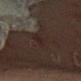Q: Was a biopsy performed?
A: imaged on a skin check; not biopsied
Q: Automated lesion metrics?
A: a footprint of about 3.5 mm², an eccentricity of roughly 0.8, and a shape-asymmetry score of about 0.3 (0 = symmetric); a lesion color around L≈19 a*≈12 b*≈14 in CIELAB, a lesion–skin lightness drop of about 5, and a normalized border contrast of about 6.5
Q: How was this image acquired?
A: ~15 mm tile from a whole-body skin photo
Q: How was the tile lit?
A: cross-polarized
Q: Lesion size?
A: about 3 mm
Q: Lesion location?
A: the right thigh
Q: Who is the patient?
A: female, in their 70s